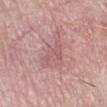Imaged with white-light lighting.
Measured at roughly 5.5 mm in maximum diameter.
A region of skin cropped from a whole-body photographic capture, roughly 15 mm wide.
A female patient, aged 73–77.
Located on the left lower leg.